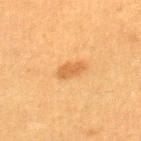The lesion was photographed on a routine skin check and not biopsied; there is no pathology result. Longest diameter approximately 3.5 mm. A female patient aged 38 to 42. The tile uses cross-polarized illumination. Located on the right thigh. The lesion-visualizer software estimated an area of roughly 4.5 mm² and two-axis asymmetry of about 0.25. Cropped from a total-body skin-imaging series; the visible field is about 15 mm.